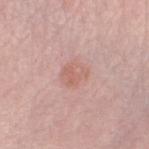  patient:
    sex: female
    age_approx: 65
  image:
    source: total-body photography crop
    field_of_view_mm: 15
  site: left thigh
  lighting: white-light